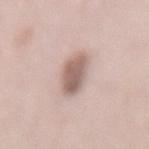Impression:
Captured during whole-body skin photography for melanoma surveillance; the lesion was not biopsied.
Background:
Longest diameter approximately 4.5 mm. The total-body-photography lesion software estimated border irregularity of about 1.5 on a 0–10 scale, internal color variation of about 4 on a 0–10 scale, and peripheral color asymmetry of about 1.5. The analysis additionally found an automated nevus-likeness rating near 85 out of 100. A female patient approximately 65 years of age. From the mid back. A 15 mm crop from a total-body photograph taken for skin-cancer surveillance.